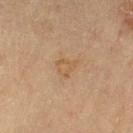No biopsy was performed on this lesion — it was imaged during a full skin examination and was not determined to be concerning.
From the right thigh.
Cropped from a whole-body photographic skin survey; the tile spans about 15 mm.
Imaged with cross-polarized lighting.
A female subject, aged approximately 80.
Approximately 2.5 mm at its widest.